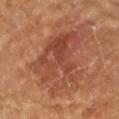Impression:
The lesion was tiled from a total-body skin photograph and was not biopsied.
Background:
This is a cross-polarized tile. From the right forearm. A close-up tile cropped from a whole-body skin photograph, about 15 mm across. A female patient in their 70s. An algorithmic analysis of the crop reported a lesion area of about 32 mm², an outline eccentricity of about 0.7 (0 = round, 1 = elongated), and a shape-asymmetry score of about 0.45 (0 = symmetric). And it measured an average lesion color of about L≈44 a*≈25 b*≈30 (CIELAB) and a normalized lesion–skin contrast near 6.5. It also reported a classifier nevus-likeness of about 0/100.